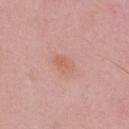Q: Was a biopsy performed?
A: imaged on a skin check; not biopsied
Q: Who is the patient?
A: male, aged approximately 50
Q: What is the imaging modality?
A: 15 mm crop, total-body photography
Q: How was the tile lit?
A: white-light illumination
Q: Lesion location?
A: the chest
Q: Lesion size?
A: about 2.5 mm